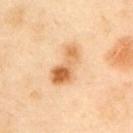Case summary:
* biopsy status: catalogued during a skin exam; not biopsied
* illumination: cross-polarized illumination
* subject: male, aged around 55
* anatomic site: the left upper arm
* image: ~15 mm tile from a whole-body skin photo